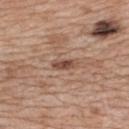Q: Is there a histopathology result?
A: total-body-photography surveillance lesion; no biopsy